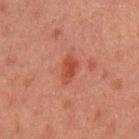biopsy_status: not biopsied; imaged during a skin examination
automated_metrics:
  area_mm2_approx: 5.0
  eccentricity: 0.8
  cielab_L: 40
  cielab_a: 27
  cielab_b: 28
  vs_skin_darker_L: 7.0
  vs_skin_contrast_norm: 7.0
  border_irregularity_0_10: 3.0
  color_variation_0_10: 2.5
  peripheral_color_asymmetry: 0.5
  nevus_likeness_0_100: 35
  lesion_detection_confidence_0_100: 100
site: left upper arm
image:
  source: total-body photography crop
  field_of_view_mm: 15
patient:
  sex: male
  age_approx: 45
lesion_size:
  long_diameter_mm_approx: 3.0